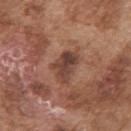  lesion_size:
    long_diameter_mm_approx: 4.0
  image:
    source: total-body photography crop
    field_of_view_mm: 15
  site: right upper arm
  lighting: white-light
  patient:
    sex: male
    age_approx: 75